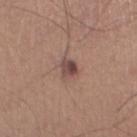image source = ~15 mm crop, total-body skin-cancer survey | subject = male, in their mid- to late 60s | location = the left thigh | automated metrics = a lesion color around L≈47 a*≈19 b*≈21 in CIELAB, a lesion–skin lightness drop of about 11, and a normalized lesion–skin contrast near 8.5 | illumination = white-light illumination | size = ~2.5 mm (longest diameter).